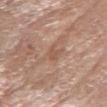<lesion>
  <biopsy_status>not biopsied; imaged during a skin examination</biopsy_status>
  <lesion_size>
    <long_diameter_mm_approx>2.5</long_diameter_mm_approx>
  </lesion_size>
  <automated_metrics>
    <color_variation_0_10>0.0</color_variation_0_10>
  </automated_metrics>
  <patient>
    <sex>female</sex>
    <age_approx>75</age_approx>
  </patient>
  <site>right forearm</site>
  <lighting>white-light</lighting>
  <image>
    <source>total-body photography crop</source>
    <field_of_view_mm>15</field_of_view_mm>
  </image>
</lesion>